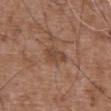Q: Is there a histopathology result?
A: imaged on a skin check; not biopsied
Q: What is the imaging modality?
A: ~15 mm tile from a whole-body skin photo
Q: Where on the body is the lesion?
A: the abdomen
Q: What are the patient's age and sex?
A: male, aged 73 to 77